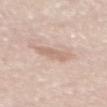Q: Is there a histopathology result?
A: catalogued during a skin exam; not biopsied
Q: What are the patient's age and sex?
A: male, aged 58 to 62
Q: What lighting was used for the tile?
A: white-light
Q: Lesion location?
A: the back
Q: What kind of image is this?
A: total-body-photography crop, ~15 mm field of view